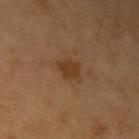Findings:
• follow-up · no biopsy performed (imaged during a skin exam)